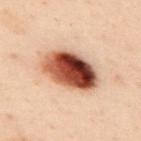This lesion was catalogued during total-body skin photography and was not selected for biopsy.
A male subject, approximately 50 years of age.
A 15 mm close-up tile from a total-body photography series done for melanoma screening.
Approximately 6.5 mm at its widest.
On the upper back.